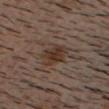{"biopsy_status": "not biopsied; imaged during a skin examination", "patient": {"sex": "male", "age_approx": 40}, "lesion_size": {"long_diameter_mm_approx": 5.0}, "image": {"source": "total-body photography crop", "field_of_view_mm": 15}, "lighting": "cross-polarized", "site": "head or neck", "automated_metrics": {"area_mm2_approx": 11.0, "eccentricity": 0.65, "shape_asymmetry": 0.35}}